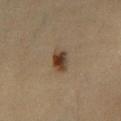Assessment: This lesion was catalogued during total-body skin photography and was not selected for biopsy. Clinical summary: Located on the left lower leg. This image is a 15 mm lesion crop taken from a total-body photograph. Captured under cross-polarized illumination. A female patient, in their 40s.